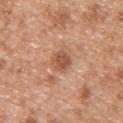| field | value |
|---|---|
| lesion diameter | ≈3 mm |
| lighting | white-light illumination |
| image source | ~15 mm tile from a whole-body skin photo |
| body site | the upper back |
| subject | male, approximately 30 years of age |